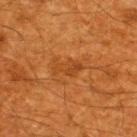Q: Was this lesion biopsied?
A: total-body-photography surveillance lesion; no biopsy
Q: What is the anatomic site?
A: the upper back
Q: What is the imaging modality?
A: 15 mm crop, total-body photography
Q: Who is the patient?
A: male, aged approximately 60